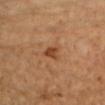follow-up = imaged on a skin check; not biopsied
diameter = ≈2.5 mm
subject = female, aged approximately 70
body site = the right upper arm
tile lighting = cross-polarized illumination
acquisition = total-body-photography crop, ~15 mm field of view